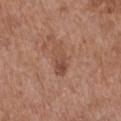<lesion>
  <biopsy_status>not biopsied; imaged during a skin examination</biopsy_status>
  <site>chest</site>
  <image>
    <source>total-body photography crop</source>
    <field_of_view_mm>15</field_of_view_mm>
  </image>
  <lighting>white-light</lighting>
  <patient>
    <sex>female</sex>
    <age_approx>75</age_approx>
  </patient>
</lesion>